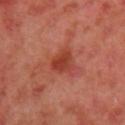| field | value |
|---|---|
| biopsy status | no biopsy performed (imaged during a skin exam) |
| lesion size | ~2.5 mm (longest diameter) |
| lighting | cross-polarized |
| automated lesion analysis | an average lesion color of about L≈42 a*≈33 b*≈33 (CIELAB), roughly 10 lightness units darker than nearby skin, and a normalized border contrast of about 8 |
| site | the left upper arm |
| imaging modality | 15 mm crop, total-body photography |
| patient | female, about 40 years old |